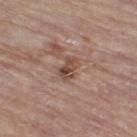Imaged during a routine full-body skin examination; the lesion was not biopsied and no histopathology is available.
A 15 mm close-up tile from a total-body photography series done for melanoma screening.
Measured at roughly 3 mm in maximum diameter.
The subject is a female roughly 70 years of age.
From the right thigh.